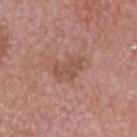<record>
<site>head or neck</site>
<automated_metrics>
  <cielab_L>51</cielab_L>
  <cielab_a>22</cielab_a>
  <cielab_b>28</cielab_b>
  <vs_skin_darker_L>7.0</vs_skin_darker_L>
  <vs_skin_contrast_norm>6.0</vs_skin_contrast_norm>
  <nevus_likeness_0_100>0</nevus_likeness_0_100>
  <lesion_detection_confidence_0_100>100</lesion_detection_confidence_0_100>
</automated_metrics>
<lesion_size>
  <long_diameter_mm_approx>4.0</long_diameter_mm_approx>
</lesion_size>
<lighting>white-light</lighting>
<patient>
  <sex>male</sex>
  <age_approx>75</age_approx>
</patient>
<image>
  <source>total-body photography crop</source>
  <field_of_view_mm>15</field_of_view_mm>
</image>
</record>